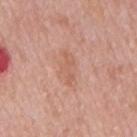Findings:
* image · ~15 mm crop, total-body skin-cancer survey
* site · the mid back
* subject · male, roughly 60 years of age
* diameter · about 4 mm
* tile lighting · white-light
* automated metrics · border irregularity of about 4 on a 0–10 scale